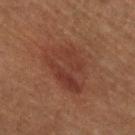The lesion was photographed on a routine skin check and not biopsied; there is no pathology result.
A 15 mm close-up tile from a total-body photography series done for melanoma screening.
Captured under cross-polarized illumination.
About 6.5 mm across.
The lesion is located on the left lower leg.
A female subject aged 78 to 82.